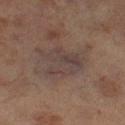notes=catalogued during a skin exam; not biopsied | acquisition=~15 mm tile from a whole-body skin photo | subject=female, aged around 60 | site=the left thigh.